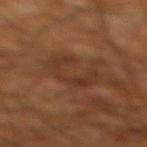Findings:
• location: the right lower leg
• imaging modality: 15 mm crop, total-body photography
• subject: male, aged 58–62